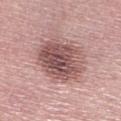workup: catalogued during a skin exam; not biopsied | lighting: white-light illumination | diameter: about 6.5 mm | subject: female, aged 63 to 67 | imaging modality: total-body-photography crop, ~15 mm field of view | anatomic site: the right lower leg.